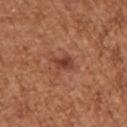The lesion was tiled from a total-body skin photograph and was not biopsied.
A female patient, aged 38 to 42.
Cropped from a total-body skin-imaging series; the visible field is about 15 mm.
Measured at roughly 3 mm in maximum diameter.
The lesion-visualizer software estimated a lesion area of about 4 mm², a shape eccentricity near 0.85, and a shape-asymmetry score of about 0.3 (0 = symmetric). And it measured a lesion color around L≈42 a*≈25 b*≈29 in CIELAB and a lesion-to-skin contrast of about 8 (normalized; higher = more distinct). The analysis additionally found a border-irregularity rating of about 3.5/10, a within-lesion color-variation index near 3/10, and radial color variation of about 1.
The lesion is located on the right upper arm.
Captured under white-light illumination.